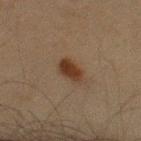Impression: Recorded during total-body skin imaging; not selected for excision or biopsy. Background: Approximately 3 mm at its widest. The subject is a male aged 58–62. From the upper back. A 15 mm crop from a total-body photograph taken for skin-cancer surveillance. An algorithmic analysis of the crop reported a footprint of about 5 mm² and a symmetry-axis asymmetry near 0.25. It also reported a lesion color around L≈27 a*≈14 b*≈24 in CIELAB and a normalized border contrast of about 10.5. The analysis additionally found border irregularity of about 2 on a 0–10 scale, internal color variation of about 2.5 on a 0–10 scale, and peripheral color asymmetry of about 1. It also reported a classifier nevus-likeness of about 100/100 and a detector confidence of about 100 out of 100 that the crop contains a lesion.